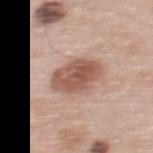The lesion was tiled from a total-body skin photograph and was not biopsied.
The recorded lesion diameter is about 5 mm.
The lesion is on the upper back.
A male patient, in their 60s.
A 15 mm crop from a total-body photograph taken for skin-cancer surveillance.
Imaged with white-light lighting.
The lesion-visualizer software estimated a footprint of about 14 mm², an outline eccentricity of about 0.6 (0 = round, 1 = elongated), and a symmetry-axis asymmetry near 0.15. The software also gave an average lesion color of about L≈55 a*≈21 b*≈27 (CIELAB) and about 13 CIELAB-L* units darker than the surrounding skin. It also reported a border-irregularity index near 1.5/10, a within-lesion color-variation index near 4.5/10, and a peripheral color-asymmetry measure near 1.5. The software also gave a classifier nevus-likeness of about 70/100 and lesion-presence confidence of about 100/100.